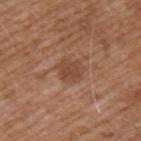Impression:
No biopsy was performed on this lesion — it was imaged during a full skin examination and was not determined to be concerning.
Clinical summary:
A lesion tile, about 15 mm wide, cut from a 3D total-body photograph. A male patient in their mid-70s. From the arm. Measured at roughly 3 mm in maximum diameter.